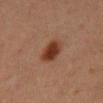| feature | finding |
|---|---|
| biopsy status | imaged on a skin check; not biopsied |
| site | the mid back |
| subject | male, aged around 60 |
| tile lighting | cross-polarized illumination |
| automated lesion analysis | a footprint of about 7 mm², a shape eccentricity near 0.8, and two-axis asymmetry of about 0.2; a lesion color around L≈33 a*≈22 b*≈28 in CIELAB and about 12 CIELAB-L* units darker than the surrounding skin; a color-variation rating of about 3/10 and radial color variation of about 1; an automated nevus-likeness rating near 100 out of 100 and a detector confidence of about 100 out of 100 that the crop contains a lesion |
| image | ~15 mm crop, total-body skin-cancer survey |
| lesion size | ≈3.5 mm |